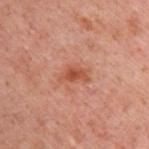Clinical impression:
The lesion was tiled from a total-body skin photograph and was not biopsied.
Acquisition and patient details:
Longest diameter approximately 3 mm. This is a cross-polarized tile. A 15 mm close-up extracted from a 3D total-body photography capture. On the upper back. The total-body-photography lesion software estimated a lesion–skin lightness drop of about 8 and a normalized border contrast of about 7.5. It also reported a border-irregularity rating of about 3/10 and a within-lesion color-variation index near 2.5/10. And it measured a lesion-detection confidence of about 100/100. The subject is a male in their 40s.